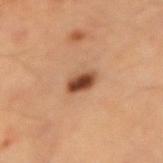Assessment:
No biopsy was performed on this lesion — it was imaged during a full skin examination and was not determined to be concerning.
Background:
This is a cross-polarized tile. The lesion is on the right forearm. A male subject about 60 years old. A 15 mm close-up tile from a total-body photography series done for melanoma screening. Automated image analysis of the tile measured a shape eccentricity near 0.75 and two-axis asymmetry of about 0.15. The software also gave a mean CIELAB color near L≈43 a*≈21 b*≈30, a lesion–skin lightness drop of about 15, and a normalized border contrast of about 11. And it measured a within-lesion color-variation index near 5.5/10 and a peripheral color-asymmetry measure near 2. It also reported a classifier nevus-likeness of about 100/100 and lesion-presence confidence of about 100/100.